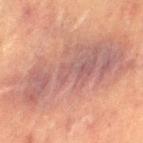A female subject aged approximately 55. The lesion is located on the left thigh. Measured at roughly 14.5 mm in maximum diameter. The tile uses cross-polarized illumination. This image is a 15 mm lesion crop taken from a total-body photograph. An algorithmic analysis of the crop reported a border-irregularity index near 5/10, a color-variation rating of about 5/10, and peripheral color asymmetry of about 1.5. And it measured a classifier nevus-likeness of about 0/100 and a detector confidence of about 90 out of 100 that the crop contains a lesion.